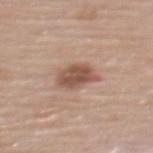notes: total-body-photography surveillance lesion; no biopsy
patient: female, about 65 years old
site: the back
tile lighting: white-light illumination
automated lesion analysis: a footprint of about 9 mm² and an outline eccentricity of about 0.7 (0 = round, 1 = elongated); a border-irregularity index near 2.5/10 and peripheral color asymmetry of about 1; a nevus-likeness score of about 85/100 and lesion-presence confidence of about 100/100
acquisition: 15 mm crop, total-body photography